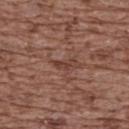| field | value |
|---|---|
| biopsy status | total-body-photography surveillance lesion; no biopsy |
| body site | the back |
| imaging modality | ~15 mm crop, total-body skin-cancer survey |
| subject | female, aged approximately 75 |
| tile lighting | white-light illumination |